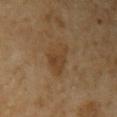Case summary:
* workup: imaged on a skin check; not biopsied
* image source: 15 mm crop, total-body photography
* anatomic site: the arm
* patient: female, aged approximately 70